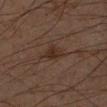Impression:
The lesion was tiled from a total-body skin photograph and was not biopsied.
Image and clinical context:
A male patient in their mid-60s. A 15 mm close-up tile from a total-body photography series done for melanoma screening. Automated image analysis of the tile measured a footprint of about 4 mm², a shape eccentricity near 0.65, and a symmetry-axis asymmetry near 0.3. And it measured a mean CIELAB color near L≈22 a*≈14 b*≈20 and a normalized lesion–skin contrast near 7.5. It also reported a border-irregularity rating of about 3/10, internal color variation of about 2 on a 0–10 scale, and a peripheral color-asymmetry measure near 0.5. The analysis additionally found a lesion-detection confidence of about 100/100. The recorded lesion diameter is about 2.5 mm. The lesion is on the left forearm. Imaged with cross-polarized lighting.